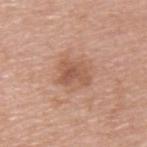Q: What are the patient's age and sex?
A: male, about 55 years old
Q: Where on the body is the lesion?
A: the upper back
Q: How was this image acquired?
A: total-body-photography crop, ~15 mm field of view
Q: Automated lesion metrics?
A: a footprint of about 7 mm² and two-axis asymmetry of about 0.35; an automated nevus-likeness rating near 15 out of 100
Q: How large is the lesion?
A: ~3.5 mm (longest diameter)
Q: How was the tile lit?
A: white-light illumination